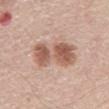No biopsy was performed on this lesion — it was imaged during a full skin examination and was not determined to be concerning. A male subject approximately 60 years of age. On the abdomen. A 15 mm crop from a total-body photograph taken for skin-cancer surveillance. Imaged with white-light lighting. About 6 mm across. The total-body-photography lesion software estimated an average lesion color of about L≈57 a*≈21 b*≈28 (CIELAB) and a lesion–skin lightness drop of about 13. The analysis additionally found a border-irregularity rating of about 4.5/10, internal color variation of about 5 on a 0–10 scale, and peripheral color asymmetry of about 2.